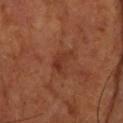notes=imaged on a skin check; not biopsied
acquisition=~15 mm tile from a whole-body skin photo
tile lighting=cross-polarized illumination
automated lesion analysis=an area of roughly 4 mm²; an average lesion color of about L≈32 a*≈24 b*≈28 (CIELAB) and about 7 CIELAB-L* units darker than the surrounding skin; a within-lesion color-variation index near 2/10 and peripheral color asymmetry of about 0.5; a classifier nevus-likeness of about 0/100 and a detector confidence of about 100 out of 100 that the crop contains a lesion
site=the upper back
lesion size=~3.5 mm (longest diameter)
subject=male, in their mid- to late 50s